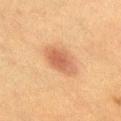The lesion was photographed on a routine skin check and not biopsied; there is no pathology result. A male subject, approximately 65 years of age. The recorded lesion diameter is about 4.5 mm. Automated tile analysis of the lesion measured an area of roughly 8 mm². It also reported a lesion color around L≈48 a*≈21 b*≈31 in CIELAB and about 9 CIELAB-L* units darker than the surrounding skin. The analysis additionally found a color-variation rating of about 3/10 and radial color variation of about 1. The lesion is on the upper back. Cropped from a total-body skin-imaging series; the visible field is about 15 mm. Captured under cross-polarized illumination.